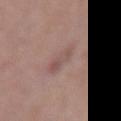{"biopsy_status": "not biopsied; imaged during a skin examination", "image": {"source": "total-body photography crop", "field_of_view_mm": 15}, "site": "right forearm", "patient": {"sex": "female", "age_approx": 40}, "lesion_size": {"long_diameter_mm_approx": 3.5}, "lighting": "white-light"}